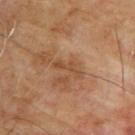Q: What is the imaging modality?
A: 15 mm crop, total-body photography
Q: Lesion size?
A: about 3.5 mm
Q: What is the anatomic site?
A: the upper back
Q: Patient demographics?
A: male, about 65 years old